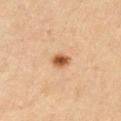<lesion>
<biopsy_status>not biopsied; imaged during a skin examination</biopsy_status>
<patient>
  <sex>male</sex>
  <age_approx>50</age_approx>
</patient>
<site>front of the torso</site>
<automated_metrics>
  <cielab_L>55</cielab_L>
  <cielab_a>22</cielab_a>
  <cielab_b>38</cielab_b>
  <vs_skin_contrast_norm>10.0</vs_skin_contrast_norm>
</automated_metrics>
<lesion_size>
  <long_diameter_mm_approx>2.5</long_diameter_mm_approx>
</lesion_size>
<image>
  <source>total-body photography crop</source>
  <field_of_view_mm>15</field_of_view_mm>
</image>
</lesion>